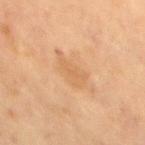The lesion was tiled from a total-body skin photograph and was not biopsied.
Longest diameter approximately 4 mm.
The total-body-photography lesion software estimated border irregularity of about 4 on a 0–10 scale, internal color variation of about 1.5 on a 0–10 scale, and radial color variation of about 0.5.
Cropped from a whole-body photographic skin survey; the tile spans about 15 mm.
Imaged with cross-polarized lighting.
The lesion is located on the chest.
The subject is a female roughly 60 years of age.